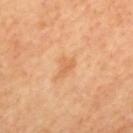The lesion was photographed on a routine skin check and not biopsied; there is no pathology result. The recorded lesion diameter is about 2.5 mm. Cropped from a total-body skin-imaging series; the visible field is about 15 mm. The lesion-visualizer software estimated an area of roughly 3 mm², an eccentricity of roughly 0.75, and a shape-asymmetry score of about 0.5 (0 = symmetric). The tile uses cross-polarized illumination. Located on the back. A male subject, aged approximately 60.